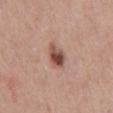Q: Was a biopsy performed?
A: total-body-photography surveillance lesion; no biopsy
Q: What is the lesion's diameter?
A: ~3.5 mm (longest diameter)
Q: What are the patient's age and sex?
A: male, roughly 60 years of age
Q: What is the imaging modality?
A: ~15 mm crop, total-body skin-cancer survey
Q: Lesion location?
A: the abdomen
Q: How was the tile lit?
A: white-light illumination